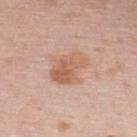Recorded during total-body skin imaging; not selected for excision or biopsy. From the upper back. A male subject, in their 40s. Cropped from a whole-body photographic skin survey; the tile spans about 15 mm. This is a white-light tile. Automated image analysis of the tile measured a footprint of about 13 mm², an eccentricity of roughly 0.65, and a symmetry-axis asymmetry near 0.3. And it measured an average lesion color of about L≈61 a*≈21 b*≈31 (CIELAB) and a normalized border contrast of about 6.5. The analysis additionally found a border-irregularity index near 3/10, internal color variation of about 6 on a 0–10 scale, and a peripheral color-asymmetry measure near 2.5. The analysis additionally found a classifier nevus-likeness of about 30/100. Measured at roughly 5 mm in maximum diameter.